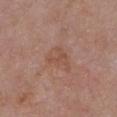Clinical impression:
This lesion was catalogued during total-body skin photography and was not selected for biopsy.
Context:
A region of skin cropped from a whole-body photographic capture, roughly 15 mm wide. Captured under white-light illumination. A female subject, aged approximately 50. About 3.5 mm across. From the front of the torso.